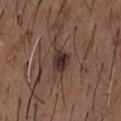Clinical impression: Recorded during total-body skin imaging; not selected for excision or biopsy. Image and clinical context: The patient is a male aged 48–52. The tile uses white-light illumination. From the chest. Cropped from a whole-body photographic skin survey; the tile spans about 15 mm.